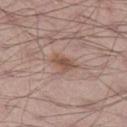Assessment:
Captured during whole-body skin photography for melanoma surveillance; the lesion was not biopsied.
Clinical summary:
A male patient, in their 70s. Located on the left thigh. A lesion tile, about 15 mm wide, cut from a 3D total-body photograph. Captured under white-light illumination.